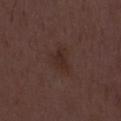Image and clinical context: A male patient, approximately 50 years of age. Measured at roughly 2.5 mm in maximum diameter. This is a white-light tile. A 15 mm close-up extracted from a 3D total-body photography capture.